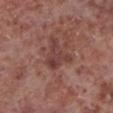notes = no biopsy performed (imaged during a skin exam)
automated metrics = an average lesion color of about L≈40 a*≈22 b*≈22 (CIELAB), about 8 CIELAB-L* units darker than the surrounding skin, and a normalized lesion–skin contrast near 6.5
image source = 15 mm crop, total-body photography
diameter = ≈4 mm
patient = male, in their mid-50s
location = the leg
illumination = white-light